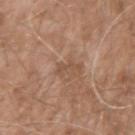Assessment: Imaged during a routine full-body skin examination; the lesion was not biopsied and no histopathology is available. Context: A male patient, approximately 60 years of age. On the right upper arm. A 15 mm crop from a total-body photograph taken for skin-cancer surveillance.